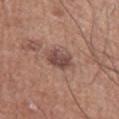Impression:
Imaged during a routine full-body skin examination; the lesion was not biopsied and no histopathology is available.
Image and clinical context:
The lesion's longest dimension is about 3 mm. The lesion-visualizer software estimated a footprint of about 7 mm², a shape eccentricity near 0.4, and two-axis asymmetry of about 0.15. It also reported a nevus-likeness score of about 35/100. A male patient aged 63 to 67. The lesion is located on the left upper arm. The tile uses white-light illumination. Cropped from a whole-body photographic skin survey; the tile spans about 15 mm.